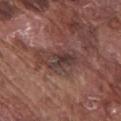biopsy status=imaged on a skin check; not biopsied | anatomic site=the chest | tile lighting=white-light illumination | image=total-body-photography crop, ~15 mm field of view | lesion diameter=about 6.5 mm | subject=male, aged approximately 75.